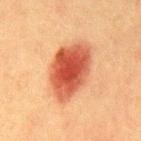Q: Was a biopsy performed?
A: total-body-photography surveillance lesion; no biopsy
Q: How was the tile lit?
A: cross-polarized
Q: Lesion size?
A: ~7 mm (longest diameter)
Q: Patient demographics?
A: male, roughly 40 years of age
Q: What kind of image is this?
A: ~15 mm crop, total-body skin-cancer survey
Q: What did automated image analysis measure?
A: a border-irregularity rating of about 2/10, a color-variation rating of about 6.5/10, and radial color variation of about 1.5; a nevus-likeness score of about 100/100 and a detector confidence of about 100 out of 100 that the crop contains a lesion
Q: What is the anatomic site?
A: the mid back